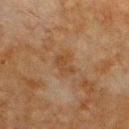Impression: The lesion was tiled from a total-body skin photograph and was not biopsied. Acquisition and patient details: Approximately 2.5 mm at its widest. The lesion is on the chest. The lesion-visualizer software estimated a lesion area of about 4.5 mm², an eccentricity of roughly 0.65, and a shape-asymmetry score of about 0.4 (0 = symmetric). And it measured a mean CIELAB color near L≈35 a*≈16 b*≈29, a lesion–skin lightness drop of about 5, and a normalized border contrast of about 6. The software also gave a border-irregularity rating of about 4/10, a within-lesion color-variation index near 1/10, and peripheral color asymmetry of about 0.5. This is a cross-polarized tile. A male subject aged around 80. A 15 mm close-up tile from a total-body photography series done for melanoma screening.